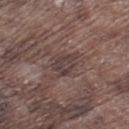Impression:
No biopsy was performed on this lesion — it was imaged during a full skin examination and was not determined to be concerning.
Image and clinical context:
The total-body-photography lesion software estimated an average lesion color of about L≈39 a*≈15 b*≈17 (CIELAB), roughly 7 lightness units darker than nearby skin, and a normalized lesion–skin contrast near 6.5. The software also gave a border-irregularity index near 3.5/10, a color-variation rating of about 2.5/10, and peripheral color asymmetry of about 1. The lesion is on the left thigh. Imaged with white-light lighting. The lesion's longest dimension is about 3.5 mm. A male patient in their mid-70s. Cropped from a whole-body photographic skin survey; the tile spans about 15 mm.